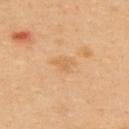{"biopsy_status": "not biopsied; imaged during a skin examination", "automated_metrics": {"nevus_likeness_0_100": 0, "lesion_detection_confidence_0_100": 100}, "site": "back", "lesion_size": {"long_diameter_mm_approx": 3.0}, "patient": {"sex": "male", "age_approx": 40}, "image": {"source": "total-body photography crop", "field_of_view_mm": 15}}